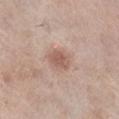| field | value |
|---|---|
| notes | no biopsy performed (imaged during a skin exam) |
| site | the leg |
| image-analysis metrics | a shape eccentricity near 0.65 and two-axis asymmetry of about 0.2; a mean CIELAB color near L≈57 a*≈20 b*≈26 and a normalized lesion–skin contrast near 7 |
| diameter | about 3 mm |
| tile lighting | white-light |
| acquisition | total-body-photography crop, ~15 mm field of view |
| patient | female, aged approximately 75 |